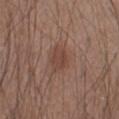workup = catalogued during a skin exam; not biopsied | image = total-body-photography crop, ~15 mm field of view | lighting = white-light | anatomic site = the right forearm | patient = male, approximately 45 years of age | automated lesion analysis = a nevus-likeness score of about 60/100 and lesion-presence confidence of about 100/100 | lesion size = about 3.5 mm.